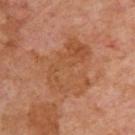Assessment:
Recorded during total-body skin imaging; not selected for excision or biopsy.
Background:
Located on the upper back. The patient is a male aged 68 to 72. About 7 mm across. The tile uses cross-polarized illumination. Cropped from a total-body skin-imaging series; the visible field is about 15 mm.